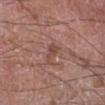| feature | finding |
|---|---|
| follow-up | imaged on a skin check; not biopsied |
| image | ~15 mm tile from a whole-body skin photo |
| lighting | white-light |
| subject | male, aged around 60 |
| diameter | about 3 mm |
| body site | the left lower leg |